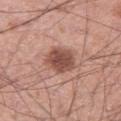Case summary:
• workup — no biopsy performed (imaged during a skin exam)
• patient — male, aged 53–57
• lesion size — about 3.5 mm
• image — 15 mm crop, total-body photography
• automated metrics — a lesion color around L≈50 a*≈23 b*≈26 in CIELAB and a normalized lesion–skin contrast near 9.5
• body site — the right thigh